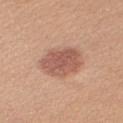From the right upper arm. Captured under white-light illumination. Approximately 4.5 mm at its widest. A 15 mm close-up extracted from a 3D total-body photography capture. A female subject, roughly 25 years of age.